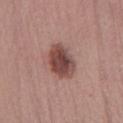{
  "biopsy_status": "not biopsied; imaged during a skin examination",
  "image": {
    "source": "total-body photography crop",
    "field_of_view_mm": 15
  },
  "site": "leg",
  "automated_metrics": {
    "cielab_L": 46,
    "cielab_a": 21,
    "cielab_b": 22,
    "vs_skin_contrast_norm": 10.5
  },
  "lighting": "white-light",
  "patient": {
    "sex": "female",
    "age_approx": 50
  }
}